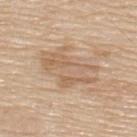{
  "biopsy_status": "not biopsied; imaged during a skin examination"
}